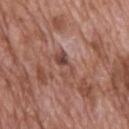The lesion was tiled from a total-body skin photograph and was not biopsied. A 15 mm close-up tile from a total-body photography series done for melanoma screening. The lesion is on the upper back. A male patient, about 70 years old.